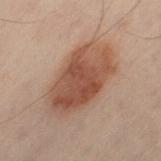Q: Was a biopsy performed?
A: imaged on a skin check; not biopsied
Q: Lesion location?
A: the left thigh
Q: What kind of image is this?
A: total-body-photography crop, ~15 mm field of view
Q: Patient demographics?
A: male, approximately 50 years of age
Q: Illumination type?
A: cross-polarized illumination
Q: Lesion size?
A: ~8.5 mm (longest diameter)